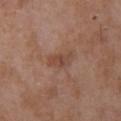image source: ~15 mm crop, total-body skin-cancer survey | anatomic site: the back | diameter: ~3 mm (longest diameter) | patient: female, aged 68–72 | tile lighting: white-light.